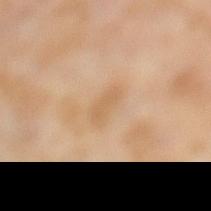Q: Is there a histopathology result?
A: imaged on a skin check; not biopsied
Q: Patient demographics?
A: female, in their mid-50s
Q: Lesion location?
A: the left lower leg
Q: How was this image acquired?
A: total-body-photography crop, ~15 mm field of view
Q: What is the lesion's diameter?
A: ≈3.5 mm
Q: How was the tile lit?
A: cross-polarized illumination